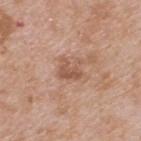notes — imaged on a skin check; not biopsied
acquisition — 15 mm crop, total-body photography
location — the back
lighting — white-light
patient — male, aged 63–67